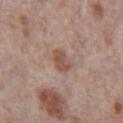No biopsy was performed on this lesion — it was imaged during a full skin examination and was not determined to be concerning. On the abdomen. A male subject in their 70s. A 15 mm close-up tile from a total-body photography series done for melanoma screening.